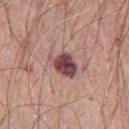The lesion was tiled from a total-body skin photograph and was not biopsied. Measured at roughly 3.5 mm in maximum diameter. Automated image analysis of the tile measured an average lesion color of about L≈45 a*≈24 b*≈17 (CIELAB) and roughly 17 lightness units darker than nearby skin. And it measured a border-irregularity index near 2.5/10 and radial color variation of about 1.5. The software also gave a nevus-likeness score of about 40/100 and a lesion-detection confidence of about 100/100. Cropped from a total-body skin-imaging series; the visible field is about 15 mm. Located on the chest. The tile uses white-light illumination. A male subject in their 60s.